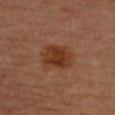follow-up = no biopsy performed (imaged during a skin exam)
location = the left upper arm
illumination = cross-polarized
acquisition = ~15 mm tile from a whole-body skin photo
image-analysis metrics = a mean CIELAB color near L≈27 a*≈19 b*≈26 and roughly 7 lightness units darker than nearby skin; a border-irregularity index near 2.5/10, a within-lesion color-variation index near 3/10, and peripheral color asymmetry of about 1; an automated nevus-likeness rating near 80 out of 100 and a detector confidence of about 100 out of 100 that the crop contains a lesion
patient = male, about 65 years old